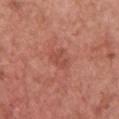notes: no biopsy performed (imaged during a skin exam)
imaging modality: total-body-photography crop, ~15 mm field of view
diameter: ~2.5 mm (longest diameter)
tile lighting: white-light illumination
location: the upper back
image-analysis metrics: an outline eccentricity of about 0.75 (0 = round, 1 = elongated) and a symmetry-axis asymmetry near 0.45; peripheral color asymmetry of about 0
subject: female, approximately 40 years of age